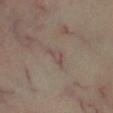Part of a total-body skin-imaging series; this lesion was reviewed on a skin check and was not flagged for biopsy.
From the right lower leg.
A male patient aged 53 to 57.
A lesion tile, about 15 mm wide, cut from a 3D total-body photograph.